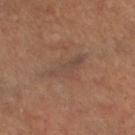Q: Was this lesion biopsied?
A: total-body-photography surveillance lesion; no biopsy
Q: Lesion size?
A: ~5.5 mm (longest diameter)
Q: Automated lesion metrics?
A: an area of roughly 8 mm², an eccentricity of roughly 0.9, and a shape-asymmetry score of about 0.35 (0 = symmetric); an automated nevus-likeness rating near 0 out of 100 and a detector confidence of about 80 out of 100 that the crop contains a lesion
Q: What are the patient's age and sex?
A: male, aged 63–67
Q: What is the imaging modality?
A: ~15 mm crop, total-body skin-cancer survey
Q: Lesion location?
A: the right lower leg